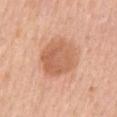Case summary:
– follow-up · catalogued during a skin exam; not biopsied
– lighting · white-light illumination
– patient · female, roughly 55 years of age
– image · 15 mm crop, total-body photography
– anatomic site · the mid back
– lesion size · ≈5.5 mm